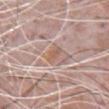follow-up — imaged on a skin check; not biopsied | imaging modality — ~15 mm tile from a whole-body skin photo | site — the chest | size — about 2.5 mm | lighting — white-light | subject — male, in their 80s.